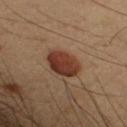notes = total-body-photography surveillance lesion; no biopsy | anatomic site = the right forearm | TBP lesion metrics = a lesion color around L≈28 a*≈19 b*≈23 in CIELAB, about 11 CIELAB-L* units darker than the surrounding skin, and a normalized lesion–skin contrast near 11.5; a classifier nevus-likeness of about 100/100 and lesion-presence confidence of about 100/100 | acquisition = ~15 mm crop, total-body skin-cancer survey | subject = male, aged approximately 55.